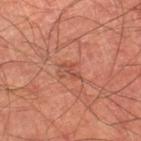<lesion>
<biopsy_status>not biopsied; imaged during a skin examination</biopsy_status>
<patient>
  <sex>male</sex>
  <age_approx>65</age_approx>
</patient>
<site>right lower leg</site>
<automated_metrics>
  <area_mm2_approx>2.0</area_mm2_approx>
  <eccentricity>0.9</eccentricity>
  <shape_asymmetry>0.35</shape_asymmetry>
  <cielab_L>49</cielab_L>
  <cielab_a>28</cielab_a>
  <cielab_b>31</cielab_b>
  <vs_skin_contrast_norm>5.5</vs_skin_contrast_norm>
</automated_metrics>
<lesion_size>
  <long_diameter_mm_approx>2.5</long_diameter_mm_approx>
</lesion_size>
<image>
  <source>total-body photography crop</source>
  <field_of_view_mm>15</field_of_view_mm>
</image>
<lighting>cross-polarized</lighting>
</lesion>